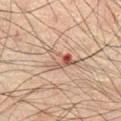Impression: Part of a total-body skin-imaging series; this lesion was reviewed on a skin check and was not flagged for biopsy. Background: Automated tile analysis of the lesion measured a border-irregularity index near 6.5/10, a color-variation rating of about 10/10, and a peripheral color-asymmetry measure near 4. And it measured a nevus-likeness score of about 0/100 and a detector confidence of about 95 out of 100 that the crop contains a lesion. Cropped from a total-body skin-imaging series; the visible field is about 15 mm. From the abdomen. A male patient aged 68 to 72.